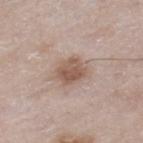Assessment: Recorded during total-body skin imaging; not selected for excision or biopsy. Image and clinical context: A male subject in their 80s. An algorithmic analysis of the crop reported a shape-asymmetry score of about 0.2 (0 = symmetric). The software also gave border irregularity of about 2 on a 0–10 scale and radial color variation of about 1. The lesion is located on the leg. A 15 mm close-up tile from a total-body photography series done for melanoma screening. Approximately 3 mm at its widest.